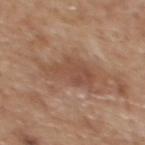Recorded during total-body skin imaging; not selected for excision or biopsy.
Approximately 6 mm at its widest.
The total-body-photography lesion software estimated a lesion color around L≈49 a*≈20 b*≈29 in CIELAB, roughly 8 lightness units darker than nearby skin, and a normalized border contrast of about 6.5. And it measured a classifier nevus-likeness of about 0/100 and a lesion-detection confidence of about 100/100.
A male patient approximately 60 years of age.
The lesion is located on the upper back.
A 15 mm close-up tile from a total-body photography series done for melanoma screening.
This is a white-light tile.